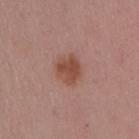  biopsy_status: not biopsied; imaged during a skin examination
  image:
    source: total-body photography crop
    field_of_view_mm: 15
  lighting: white-light
  patient:
    sex: female
    age_approx: 45
  lesion_size:
    long_diameter_mm_approx: 3.5
  site: right upper arm